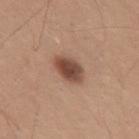Assessment:
Part of a total-body skin-imaging series; this lesion was reviewed on a skin check and was not flagged for biopsy.
Clinical summary:
A male subject in their 30s. A 15 mm crop from a total-body photograph taken for skin-cancer surveillance. From the mid back. Automated image analysis of the tile measured a lesion color around L≈47 a*≈21 b*≈27 in CIELAB, roughly 14 lightness units darker than nearby skin, and a lesion-to-skin contrast of about 10 (normalized; higher = more distinct). The analysis additionally found a border-irregularity rating of about 1.5/10, a color-variation rating of about 4.5/10, and a peripheral color-asymmetry measure near 1.5. Imaged with white-light lighting.